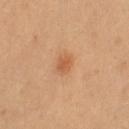The lesion was tiled from a total-body skin photograph and was not biopsied.